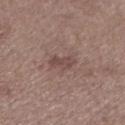Located on the left lower leg. The patient is a female approximately 65 years of age. About 3.5 mm across. A 15 mm crop from a total-body photograph taken for skin-cancer surveillance.